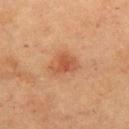notes: catalogued during a skin exam; not biopsied
lesion diameter: ~3.5 mm (longest diameter)
TBP lesion metrics: a lesion area of about 6 mm², a shape eccentricity near 0.7, and a symmetry-axis asymmetry near 0.4; a border-irregularity index near 4/10, internal color variation of about 2.5 on a 0–10 scale, and peripheral color asymmetry of about 0.5; a nevus-likeness score of about 55/100 and a detector confidence of about 100 out of 100 that the crop contains a lesion
body site: the chest
image: 15 mm crop, total-body photography
lighting: cross-polarized illumination
subject: female, aged around 60